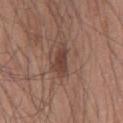biopsy status: imaged on a skin check; not biopsied
site: the chest
acquisition: 15 mm crop, total-body photography
size: ~4 mm (longest diameter)
image-analysis metrics: a footprint of about 6.5 mm², a shape eccentricity near 0.85, and a symmetry-axis asymmetry near 0.35; a nevus-likeness score of about 80/100 and a lesion-detection confidence of about 100/100
subject: male, aged approximately 55